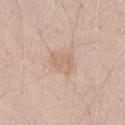Findings:
- workup · total-body-photography surveillance lesion; no biopsy
- size · ≈3.5 mm
- site · the right thigh
- subject · male, aged 43–47
- automated metrics · a footprint of about 7 mm², an eccentricity of roughly 0.7, and two-axis asymmetry of about 0.3
- acquisition · total-body-photography crop, ~15 mm field of view